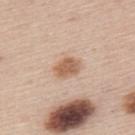A 15 mm crop from a total-body photograph taken for skin-cancer surveillance. Located on the back. This is a white-light tile. The recorded lesion diameter is about 3 mm. The patient is a male aged around 45. Automated image analysis of the tile measured a footprint of about 6 mm² and a symmetry-axis asymmetry near 0.2. The analysis additionally found border irregularity of about 1.5 on a 0–10 scale, a within-lesion color-variation index near 2/10, and a peripheral color-asymmetry measure near 0.5. The analysis additionally found a detector confidence of about 100 out of 100 that the crop contains a lesion.